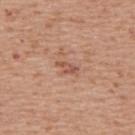Clinical impression:
The lesion was photographed on a routine skin check and not biopsied; there is no pathology result.
Background:
The patient is a male in their 70s. Captured under white-light illumination. The lesion is located on the upper back. The recorded lesion diameter is about 2.5 mm. Automated tile analysis of the lesion measured a mean CIELAB color near L≈54 a*≈24 b*≈29, roughly 9 lightness units darker than nearby skin, and a normalized lesion–skin contrast near 6.5. The software also gave a border-irregularity rating of about 4/10, a within-lesion color-variation index near 0/10, and peripheral color asymmetry of about 0. And it measured a lesion-detection confidence of about 100/100. A lesion tile, about 15 mm wide, cut from a 3D total-body photograph.